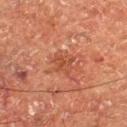Assessment:
No biopsy was performed on this lesion — it was imaged during a full skin examination and was not determined to be concerning.
Context:
A male subject aged 73 to 77. Captured under cross-polarized illumination. Located on the right thigh. Cropped from a whole-body photographic skin survey; the tile spans about 15 mm. The lesion's longest dimension is about 3 mm.